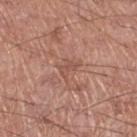The lesion was tiled from a total-body skin photograph and was not biopsied. A male patient aged around 65. On the right thigh. The lesion-visualizer software estimated an eccentricity of roughly 0.75 and a shape-asymmetry score of about 0.45 (0 = symmetric). And it measured a lesion color around L≈52 a*≈22 b*≈27 in CIELAB, about 7 CIELAB-L* units darker than the surrounding skin, and a normalized lesion–skin contrast near 5. The analysis additionally found a classifier nevus-likeness of about 0/100. A close-up tile cropped from a whole-body skin photograph, about 15 mm across.